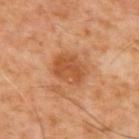Captured during whole-body skin photography for melanoma surveillance; the lesion was not biopsied. A 15 mm close-up tile from a total-body photography series done for melanoma screening. A male subject, roughly 60 years of age. From the upper back. Automated image analysis of the tile measured a lesion area of about 11 mm² and a symmetry-axis asymmetry near 0.15. And it measured an average lesion color of about L≈49 a*≈25 b*≈37 (CIELAB) and about 9 CIELAB-L* units darker than the surrounding skin. The analysis additionally found a nevus-likeness score of about 70/100 and lesion-presence confidence of about 100/100. The tile uses cross-polarized illumination.